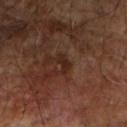Part of a total-body skin-imaging series; this lesion was reviewed on a skin check and was not flagged for biopsy.